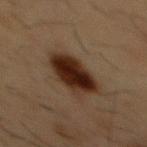Impression:
Part of a total-body skin-imaging series; this lesion was reviewed on a skin check and was not flagged for biopsy.
Clinical summary:
The lesion is located on the front of the torso. Automated image analysis of the tile measured a border-irregularity index near 2/10, a within-lesion color-variation index near 4.5/10, and peripheral color asymmetry of about 1.5. A 15 mm close-up tile from a total-body photography series done for melanoma screening. The patient is a male in their mid- to late 50s. Longest diameter approximately 5 mm. This is a cross-polarized tile.